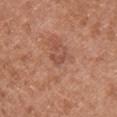Imaged during a routine full-body skin examination; the lesion was not biopsied and no histopathology is available. A 15 mm close-up extracted from a 3D total-body photography capture. Automated image analysis of the tile measured a lesion color around L≈50 a*≈24 b*≈29 in CIELAB, a lesion–skin lightness drop of about 7, and a normalized lesion–skin contrast near 5. A female subject aged 38–42. Located on the left upper arm. The recorded lesion diameter is about 2.5 mm.